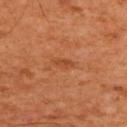Findings:
* notes — total-body-photography surveillance lesion; no biopsy
* diameter — ≈2.5 mm
* imaging modality — total-body-photography crop, ~15 mm field of view
* lighting — cross-polarized illumination
* TBP lesion metrics — a lesion color around L≈45 a*≈28 b*≈38 in CIELAB and a lesion-to-skin contrast of about 5.5 (normalized; higher = more distinct); a border-irregularity rating of about 3.5/10, a color-variation rating of about 0/10, and radial color variation of about 0
* anatomic site — the upper back
* subject — male, in their mid- to late 60s